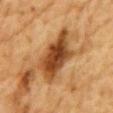Q: Was this lesion biopsied?
A: total-body-photography surveillance lesion; no biopsy
Q: Lesion location?
A: the front of the torso
Q: Patient demographics?
A: male, approximately 85 years of age or older
Q: Illumination type?
A: cross-polarized illumination
Q: What kind of image is this?
A: ~15 mm tile from a whole-body skin photo
Q: What is the lesion's diameter?
A: ≈7 mm